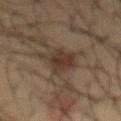notes: total-body-photography surveillance lesion; no biopsy | TBP lesion metrics: an area of roughly 9 mm², a shape eccentricity near 0.8, and a shape-asymmetry score of about 0.35 (0 = symmetric); a border-irregularity rating of about 4.5/10 and a within-lesion color-variation index near 3.5/10; an automated nevus-likeness rating near 40 out of 100 and lesion-presence confidence of about 95/100 | lesion diameter: about 4.5 mm | lighting: cross-polarized | image source: ~15 mm tile from a whole-body skin photo | body site: the abdomen | subject: male, approximately 35 years of age.